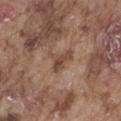{"biopsy_status": "not biopsied; imaged during a skin examination", "site": "abdomen", "image": {"source": "total-body photography crop", "field_of_view_mm": 15}, "patient": {"sex": "male", "age_approx": 75}}